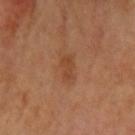Impression: Part of a total-body skin-imaging series; this lesion was reviewed on a skin check and was not flagged for biopsy. Background: This is a cross-polarized tile. On the left upper arm. About 3 mm across. This image is a 15 mm lesion crop taken from a total-body photograph. A female patient in their mid-60s.